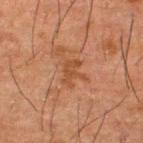{
  "biopsy_status": "not biopsied; imaged during a skin examination",
  "site": "upper back",
  "automated_metrics": {
    "cielab_L": 37,
    "cielab_a": 20,
    "cielab_b": 30,
    "vs_skin_contrast_norm": 6.0,
    "nevus_likeness_0_100": 0,
    "lesion_detection_confidence_0_100": 100
  },
  "image": {
    "source": "total-body photography crop",
    "field_of_view_mm": 15
  },
  "patient": {
    "sex": "male",
    "age_approx": 50
  }
}